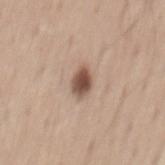Captured during whole-body skin photography for melanoma surveillance; the lesion was not biopsied.
Approximately 3 mm at its widest.
A roughly 15 mm field-of-view crop from a total-body skin photograph.
On the mid back.
A male patient aged around 45.
Captured under white-light illumination.